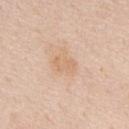Q: Was a biopsy performed?
A: imaged on a skin check; not biopsied
Q: Lesion size?
A: about 3 mm
Q: Patient demographics?
A: male, aged approximately 60
Q: What lighting was used for the tile?
A: white-light
Q: How was this image acquired?
A: ~15 mm tile from a whole-body skin photo
Q: Where on the body is the lesion?
A: the mid back